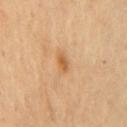{"biopsy_status": "not biopsied; imaged during a skin examination", "image": {"source": "total-body photography crop", "field_of_view_mm": 15}, "patient": {"sex": "male", "age_approx": 70}, "site": "chest", "lighting": "cross-polarized", "automated_metrics": {"area_mm2_approx": 3.0, "cielab_L": 56, "cielab_a": 20, "cielab_b": 39, "vs_skin_darker_L": 9.0}, "lesion_size": {"long_diameter_mm_approx": 2.5}}